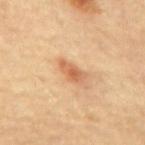<tbp_lesion>
<lighting>cross-polarized</lighting>
<patient>
  <sex>male</sex>
  <age_approx>85+</age_approx>
</patient>
<site>mid back</site>
<lesion_size>
  <long_diameter_mm_approx>3.5</long_diameter_mm_approx>
</lesion_size>
<automated_metrics>
  <nevus_likeness_0_100>45</nevus_likeness_0_100>
  <lesion_detection_confidence_0_100>100</lesion_detection_confidence_0_100>
</automated_metrics>
<image>
  <source>total-body photography crop</source>
  <field_of_view_mm>15</field_of_view_mm>
</image>
</tbp_lesion>